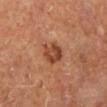Captured during whole-body skin photography for melanoma surveillance; the lesion was not biopsied. From the right lower leg. A close-up tile cropped from a whole-body skin photograph, about 15 mm across. The subject is female. About 3.5 mm across. Automated image analysis of the tile measured a classifier nevus-likeness of about 60/100 and a lesion-detection confidence of about 100/100. Imaged with cross-polarized lighting.